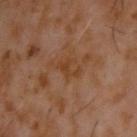• workup · catalogued during a skin exam; not biopsied
• site · the back
• image source · ~15 mm crop, total-body skin-cancer survey
• patient · male, about 60 years old
• diameter · ~3 mm (longest diameter)
• illumination · cross-polarized illumination
• automated metrics · a lesion-to-skin contrast of about 6 (normalized; higher = more distinct); a border-irregularity index near 6.5/10, a color-variation rating of about 0/10, and a peripheral color-asymmetry measure near 0; a classifier nevus-likeness of about 0/100 and a lesion-detection confidence of about 100/100